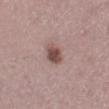<record>
  <lesion_size>
    <long_diameter_mm_approx>3.0</long_diameter_mm_approx>
  </lesion_size>
  <patient>
    <sex>female</sex>
    <age_approx>40</age_approx>
  </patient>
  <image>
    <source>total-body photography crop</source>
    <field_of_view_mm>15</field_of_view_mm>
  </image>
  <site>leg</site>
</record>